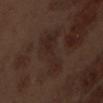notes: no biopsy performed (imaged during a skin exam) | body site: the abdomen | acquisition: ~15 mm crop, total-body skin-cancer survey | subject: male, in their 70s.